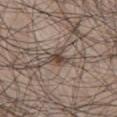Part of a total-body skin-imaging series; this lesion was reviewed on a skin check and was not flagged for biopsy.
On the front of the torso.
A 15 mm crop from a total-body photograph taken for skin-cancer surveillance.
The subject is a male aged 48 to 52.